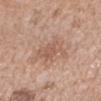biopsy_status: not biopsied; imaged during a skin examination
site: right forearm
image:
  source: total-body photography crop
  field_of_view_mm: 15
patient:
  sex: female
  age_approx: 60
lesion_size:
  long_diameter_mm_approx: 4.0